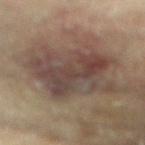{"biopsy_status": "not biopsied; imaged during a skin examination", "automated_metrics": {"cielab_L": 38, "cielab_a": 13, "cielab_b": 19, "vs_skin_darker_L": 9.0, "vs_skin_contrast_norm": 8.5, "border_irregularity_0_10": 6.5, "peripheral_color_asymmetry": 2.0}, "site": "right arm", "lesion_size": {"long_diameter_mm_approx": 8.0}, "lighting": "cross-polarized", "patient": {"sex": "female", "age_approx": 80}, "image": {"source": "total-body photography crop", "field_of_view_mm": 15}}